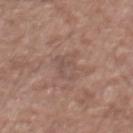Impression:
Captured during whole-body skin photography for melanoma surveillance; the lesion was not biopsied.